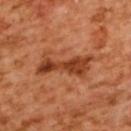  biopsy_status: not biopsied; imaged during a skin examination
  lighting: cross-polarized
  lesion_size:
    long_diameter_mm_approx: 6.5
  site: upper back
  patient:
    sex: female
    age_approx: 55
  automated_metrics:
    lesion_detection_confidence_0_100: 100
  image:
    source: total-body photography crop
    field_of_view_mm: 15